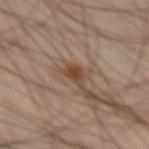biopsy status = catalogued during a skin exam; not biopsied
lesion size = ≈3 mm
image = total-body-photography crop, ~15 mm field of view
body site = the abdomen
subject = male, aged 58 to 62